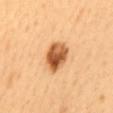site — the back | subject — female, in their mid-50s | image source — ~15 mm tile from a whole-body skin photo | illumination — cross-polarized illumination | diameter — ~4.5 mm (longest diameter) | automated metrics — an area of roughly 10 mm², a shape eccentricity near 0.7, and two-axis asymmetry of about 0.1; an average lesion color of about L≈59 a*≈26 b*≈43 (CIELAB), roughly 19 lightness units darker than nearby skin, and a normalized border contrast of about 11; a classifier nevus-likeness of about 100/100.